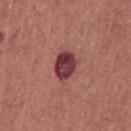biopsy status = catalogued during a skin exam; not biopsied
image = ~15 mm tile from a whole-body skin photo
site = the chest
subject = male, roughly 65 years of age